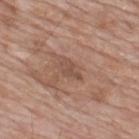biopsy_status: not biopsied; imaged during a skin examination
lighting: white-light
automated_metrics:
  area_mm2_approx: 3.5
  shape_asymmetry: 0.35
  cielab_L: 50
  cielab_a: 19
  cielab_b: 26
  vs_skin_contrast_norm: 5.5
  border_irregularity_0_10: 3.5
  color_variation_0_10: 1.5
  peripheral_color_asymmetry: 0.5
patient:
  sex: male
  age_approx: 60
site: back
lesion_size:
  long_diameter_mm_approx: 3.0
image:
  source: total-body photography crop
  field_of_view_mm: 15